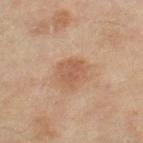Imaged during a routine full-body skin examination; the lesion was not biopsied and no histopathology is available. The patient is a female aged approximately 60. From the leg. A 15 mm crop from a total-body photograph taken for skin-cancer surveillance. Captured under cross-polarized illumination. The total-body-photography lesion software estimated a lesion color around L≈50 a*≈20 b*≈29 in CIELAB, about 7 CIELAB-L* units darker than the surrounding skin, and a normalized lesion–skin contrast near 5.5. The software also gave a within-lesion color-variation index near 2.5/10 and radial color variation of about 1. Longest diameter approximately 3 mm.